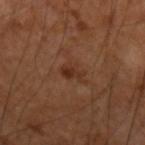Part of a total-body skin-imaging series; this lesion was reviewed on a skin check and was not flagged for biopsy. A 15 mm close-up tile from a total-body photography series done for melanoma screening. From the left forearm. A male patient aged 53 to 57. Imaged with cross-polarized lighting.